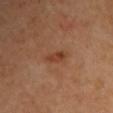No biopsy was performed on this lesion — it was imaged during a full skin examination and was not determined to be concerning.
An algorithmic analysis of the crop reported an area of roughly 3 mm², a shape eccentricity near 0.85, and a shape-asymmetry score of about 0.2 (0 = symmetric). The software also gave a mean CIELAB color near L≈40 a*≈23 b*≈33 and a lesion-to-skin contrast of about 7 (normalized; higher = more distinct).
A female patient, aged 38–42.
This is a cross-polarized tile.
About 2.5 mm across.
On the head or neck.
Cropped from a whole-body photographic skin survey; the tile spans about 15 mm.